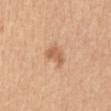Recorded during total-body skin imaging; not selected for excision or biopsy. A female patient, in their mid- to late 30s. This is a white-light tile. Cropped from a total-body skin-imaging series; the visible field is about 15 mm. An algorithmic analysis of the crop reported a shape eccentricity near 0.75 and two-axis asymmetry of about 0.4. The lesion is on the abdomen.